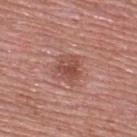Part of a total-body skin-imaging series; this lesion was reviewed on a skin check and was not flagged for biopsy. A close-up tile cropped from a whole-body skin photograph, about 15 mm across. Longest diameter approximately 3 mm. On the upper back. Imaged with white-light lighting. The subject is a male roughly 50 years of age.